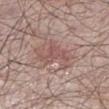workup: imaged on a skin check; not biopsied
automated metrics: a shape eccentricity near 0.75 and a symmetry-axis asymmetry near 0.6; border irregularity of about 7.5 on a 0–10 scale, a within-lesion color-variation index near 2/10, and peripheral color asymmetry of about 0.5
patient: male, aged approximately 55
acquisition: 15 mm crop, total-body photography
lesion diameter: about 4 mm
body site: the leg